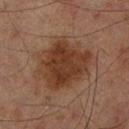This lesion was catalogued during total-body skin photography and was not selected for biopsy. The subject is a male approximately 70 years of age. Cropped from a whole-body photographic skin survey; the tile spans about 15 mm. An algorithmic analysis of the crop reported a mean CIELAB color near L≈29 a*≈17 b*≈25, a lesion–skin lightness drop of about 9, and a normalized border contrast of about 9. And it measured a color-variation rating of about 3.5/10 and peripheral color asymmetry of about 1. The software also gave a classifier nevus-likeness of about 70/100 and a lesion-detection confidence of about 100/100. The tile uses cross-polarized illumination. The recorded lesion diameter is about 6.5 mm. The lesion is on the right lower leg.